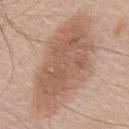Part of a total-body skin-imaging series; this lesion was reviewed on a skin check and was not flagged for biopsy.
Imaged with white-light lighting.
A region of skin cropped from a whole-body photographic capture, roughly 15 mm wide.
A male patient aged 58–62.
The lesion is on the chest.
The lesion's longest dimension is about 12.5 mm.
The total-body-photography lesion software estimated a lesion area of about 55 mm², a shape eccentricity near 0.85, and a shape-asymmetry score of about 0.15 (0 = symmetric). And it measured an average lesion color of about L≈58 a*≈19 b*≈28 (CIELAB), a lesion–skin lightness drop of about 10, and a normalized border contrast of about 7. And it measured a classifier nevus-likeness of about 50/100 and a lesion-detection confidence of about 100/100.